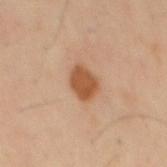Q: Is there a histopathology result?
A: total-body-photography surveillance lesion; no biopsy
Q: Patient demographics?
A: male, aged approximately 40
Q: What is the lesion's diameter?
A: ≈3 mm
Q: Lesion location?
A: the mid back
Q: What lighting was used for the tile?
A: cross-polarized illumination
Q: Automated lesion metrics?
A: a footprint of about 7 mm² and an outline eccentricity of about 0.6 (0 = round, 1 = elongated); an average lesion color of about L≈52 a*≈23 b*≈35 (CIELAB) and a normalized lesion–skin contrast near 9.5; a peripheral color-asymmetry measure near 0.5; a nevus-likeness score of about 100/100 and lesion-presence confidence of about 100/100
Q: How was this image acquired?
A: total-body-photography crop, ~15 mm field of view